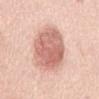Q: Is there a histopathology result?
A: total-body-photography surveillance lesion; no biopsy
Q: Who is the patient?
A: female, aged 63 to 67
Q: Illumination type?
A: white-light
Q: Lesion location?
A: the abdomen
Q: How large is the lesion?
A: ≈7.5 mm
Q: What is the imaging modality?
A: ~15 mm tile from a whole-body skin photo
Q: Automated lesion metrics?
A: a footprint of about 26 mm², an eccentricity of roughly 0.8, and a shape-asymmetry score of about 0.1 (0 = symmetric); a lesion color around L≈66 a*≈24 b*≈27 in CIELAB, a lesion–skin lightness drop of about 14, and a normalized border contrast of about 8